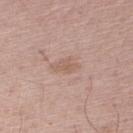Recorded during total-body skin imaging; not selected for excision or biopsy. Imaged with white-light lighting. The lesion is on the right upper arm. A 15 mm crop from a total-body photograph taken for skin-cancer surveillance. A male patient about 55 years old. Approximately 2.5 mm at its widest.